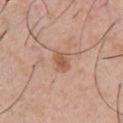Imaged during a routine full-body skin examination; the lesion was not biopsied and no histopathology is available.